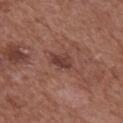  biopsy_status: not biopsied; imaged during a skin examination
  patient:
    sex: male
    age_approx: 75
  site: front of the torso
  image:
    source: total-body photography crop
    field_of_view_mm: 15